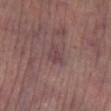No biopsy was performed on this lesion — it was imaged during a full skin examination and was not determined to be concerning.